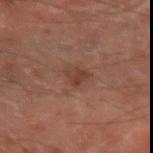- follow-up: imaged on a skin check; not biopsied
- illumination: cross-polarized
- anatomic site: the right forearm
- subject: male, roughly 70 years of age
- diameter: about 3 mm
- image: 15 mm crop, total-body photography
- automated lesion analysis: a classifier nevus-likeness of about 15/100 and a detector confidence of about 100 out of 100 that the crop contains a lesion